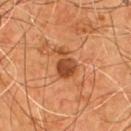Q: What is the imaging modality?
A: 15 mm crop, total-body photography
Q: Lesion location?
A: the chest
Q: How was the tile lit?
A: cross-polarized
Q: Who is the patient?
A: male, roughly 55 years of age
Q: What is the lesion's diameter?
A: ≈3.5 mm